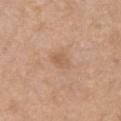workup: imaged on a skin check; not biopsied | size: ≈2.5 mm | lighting: white-light illumination | patient: male, aged 68–72 | acquisition: total-body-photography crop, ~15 mm field of view | location: the chest | automated metrics: a footprint of about 4 mm², a shape eccentricity near 0.75, and a shape-asymmetry score of about 0.2 (0 = symmetric); a normalized lesion–skin contrast near 5.